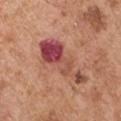follow-up: no biopsy performed (imaged during a skin exam) | location: the left upper arm | image-analysis metrics: a footprint of about 16 mm² and an outline eccentricity of about 0.95 (0 = round, 1 = elongated); a mean CIELAB color near L≈48 a*≈29 b*≈26, roughly 13 lightness units darker than nearby skin, and a normalized lesion–skin contrast near 10 | image source: total-body-photography crop, ~15 mm field of view | subject: male, in their mid- to late 50s.